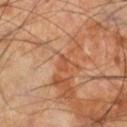Clinical impression: Recorded during total-body skin imaging; not selected for excision or biopsy. Background: The lesion is on the right lower leg. A male patient, about 55 years old. This image is a 15 mm lesion crop taken from a total-body photograph.